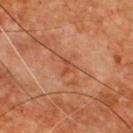biopsy_status: not biopsied; imaged during a skin examination
automated_metrics:
  eccentricity: 0.85
  shape_asymmetry: 0.55
image:
  source: total-body photography crop
  field_of_view_mm: 15
lesion_size:
  long_diameter_mm_approx: 2.0
patient:
  sex: male
  age_approx: 55
site: front of the torso